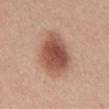  biopsy_status: not biopsied; imaged during a skin examination
  lesion_size:
    long_diameter_mm_approx: 6.0
  image:
    source: total-body photography crop
    field_of_view_mm: 15
  automated_metrics:
    cielab_L: 53
    cielab_a: 23
    cielab_b: 28
    vs_skin_darker_L: 14.0
    vs_skin_contrast_norm: 9.5
    color_variation_0_10: 5.5
    peripheral_color_asymmetry: 1.5
  site: abdomen
  patient:
    sex: male
    age_approx: 55
  lighting: white-light